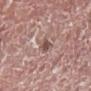Recorded during total-body skin imaging; not selected for excision or biopsy.
This is a white-light tile.
A male patient, aged 73 to 77.
A roughly 15 mm field-of-view crop from a total-body skin photograph.
On the right lower leg.